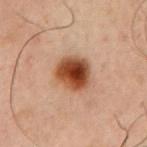Acquisition and patient details:
This image is a 15 mm lesion crop taken from a total-body photograph. The recorded lesion diameter is about 4 mm. From the front of the torso. A male patient roughly 60 years of age.